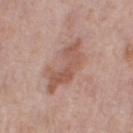Captured during whole-body skin photography for melanoma surveillance; the lesion was not biopsied. A 15 mm crop from a total-body photograph taken for skin-cancer surveillance. A female patient, aged 48 to 52. On the right thigh.